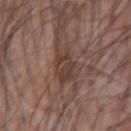Imaged during a routine full-body skin examination; the lesion was not biopsied and no histopathology is available. A region of skin cropped from a whole-body photographic capture, roughly 15 mm wide. Located on the left forearm. A male patient aged around 60.